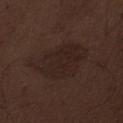imaging modality = ~15 mm crop, total-body skin-cancer survey
subject = male, roughly 70 years of age
body site = the abdomen
lesion diameter = ≈7.5 mm
automated lesion analysis = a lesion area of about 22 mm² and two-axis asymmetry of about 0.25